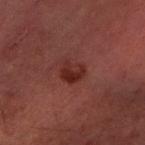Context:
Located on the right forearm. A region of skin cropped from a whole-body photographic capture, roughly 15 mm wide. A male patient, aged 58–62.